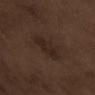Located on the arm. About 3.5 mm across. This image is a 15 mm lesion crop taken from a total-body photograph. The tile uses white-light illumination. A male patient in their 70s.